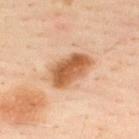Q: Is there a histopathology result?
A: catalogued during a skin exam; not biopsied